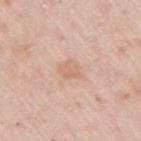body site = the right upper arm
subject = female, in their mid- to late 60s
acquisition = total-body-photography crop, ~15 mm field of view
illumination = white-light illumination